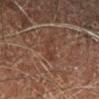Part of a total-body skin-imaging series; this lesion was reviewed on a skin check and was not flagged for biopsy.